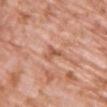Q: Was a biopsy performed?
A: total-body-photography surveillance lesion; no biopsy
Q: Who is the patient?
A: female, aged approximately 70
Q: Lesion location?
A: the upper back
Q: What is the imaging modality?
A: 15 mm crop, total-body photography
Q: How was the tile lit?
A: white-light